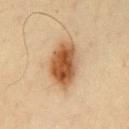Clinical impression: No biopsy was performed on this lesion — it was imaged during a full skin examination and was not determined to be concerning. Context: Longest diameter approximately 6.5 mm. This is a cross-polarized tile. Located on the front of the torso. A male subject approximately 65 years of age. A 15 mm crop from a total-body photograph taken for skin-cancer surveillance.